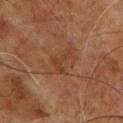Captured during whole-body skin photography for melanoma surveillance; the lesion was not biopsied. This is a cross-polarized tile. A male subject, in their 60s. On the chest. A lesion tile, about 15 mm wide, cut from a 3D total-body photograph. The lesion's longest dimension is about 3.5 mm.